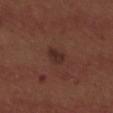Imaged during a routine full-body skin examination; the lesion was not biopsied and no histopathology is available.
Approximately 2.5 mm at its widest.
From the upper back.
An algorithmic analysis of the crop reported an area of roughly 3.5 mm², an outline eccentricity of about 0.8 (0 = round, 1 = elongated), and two-axis asymmetry of about 0.2. And it measured a border-irregularity rating of about 2/10 and a color-variation rating of about 2/10.
The patient is a male approximately 30 years of age.
The tile uses white-light illumination.
A roughly 15 mm field-of-view crop from a total-body skin photograph.